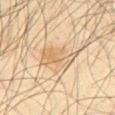Imaged during a routine full-body skin examination; the lesion was not biopsied and no histopathology is available. A lesion tile, about 15 mm wide, cut from a 3D total-body photograph. Located on the abdomen. The patient is a male about 65 years old.